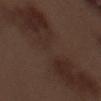Impression: Part of a total-body skin-imaging series; this lesion was reviewed on a skin check and was not flagged for biopsy. Acquisition and patient details: On the left forearm. Captured under white-light illumination. A male patient aged 68 to 72. Longest diameter approximately 17 mm. A roughly 15 mm field-of-view crop from a total-body skin photograph.